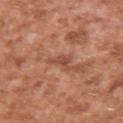notes = no biopsy performed (imaged during a skin exam)
image source = 15 mm crop, total-body photography
image-analysis metrics = a nevus-likeness score of about 0/100
anatomic site = the right upper arm
tile lighting = white-light
lesion diameter = ~3.5 mm (longest diameter)
subject = male, roughly 45 years of age